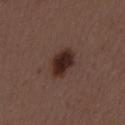follow-up — imaged on a skin check; not biopsied
location — the mid back
subject — female, about 50 years old
image — total-body-photography crop, ~15 mm field of view